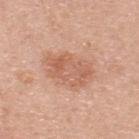Assessment:
Part of a total-body skin-imaging series; this lesion was reviewed on a skin check and was not flagged for biopsy.